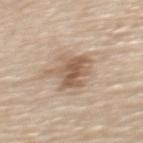Q: Is there a histopathology result?
A: imaged on a skin check; not biopsied
Q: How large is the lesion?
A: ~5.5 mm (longest diameter)
Q: How was this image acquired?
A: 15 mm crop, total-body photography
Q: Who is the patient?
A: female, aged 63–67
Q: What lighting was used for the tile?
A: white-light illumination
Q: Automated lesion metrics?
A: an area of roughly 13 mm² and a shape-asymmetry score of about 0.35 (0 = symmetric); a lesion color around L≈58 a*≈16 b*≈29 in CIELAB, about 12 CIELAB-L* units darker than the surrounding skin, and a normalized lesion–skin contrast near 7.5; a within-lesion color-variation index near 5/10 and peripheral color asymmetry of about 2
Q: What is the anatomic site?
A: the back